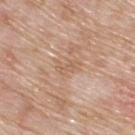Assessment:
Captured during whole-body skin photography for melanoma surveillance; the lesion was not biopsied.
Background:
A 15 mm crop from a total-body photograph taken for skin-cancer surveillance. Approximately 3 mm at its widest. Located on the upper back. A male patient, roughly 60 years of age. Captured under white-light illumination.